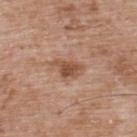<record>
  <site>upper back</site>
  <patient>
    <sex>male</sex>
    <age_approx>50</age_approx>
  </patient>
  <image>
    <source>total-body photography crop</source>
    <field_of_view_mm>15</field_of_view_mm>
  </image>
  <lesion_size>
    <long_diameter_mm_approx>3.5</long_diameter_mm_approx>
  </lesion_size>
  <lighting>white-light</lighting>
  <automated_metrics>
    <border_irregularity_0_10>4.0</border_irregularity_0_10>
    <color_variation_0_10>3.0</color_variation_0_10>
    <peripheral_color_asymmetry>1.0</peripheral_color_asymmetry>
  </automated_metrics>
</record>